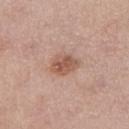Recorded during total-body skin imaging; not selected for excision or biopsy. Located on the left thigh. A 15 mm close-up tile from a total-body photography series done for melanoma screening. The tile uses white-light illumination. Approximately 3.5 mm at its widest. A female patient aged around 55.